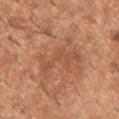Clinical impression:
Part of a total-body skin-imaging series; this lesion was reviewed on a skin check and was not flagged for biopsy.
Clinical summary:
The lesion is on the chest. The subject is a male approximately 40 years of age. A 15 mm crop from a total-body photograph taken for skin-cancer surveillance. The total-body-photography lesion software estimated a within-lesion color-variation index near 1.5/10 and a peripheral color-asymmetry measure near 0.5.